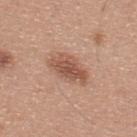Captured during whole-body skin photography for melanoma surveillance; the lesion was not biopsied. The tile uses white-light illumination. Located on the upper back. A male subject, approximately 30 years of age. A region of skin cropped from a whole-body photographic capture, roughly 15 mm wide.